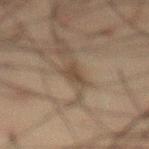{"biopsy_status": "not biopsied; imaged during a skin examination", "lesion_size": {"long_diameter_mm_approx": 3.0}, "patient": {"sex": "male", "age_approx": 60}, "image": {"source": "total-body photography crop", "field_of_view_mm": 15}, "automated_metrics": {"area_mm2_approx": 3.5, "eccentricity": 0.85, "shape_asymmetry": 0.45, "cielab_L": 34, "cielab_a": 11, "cielab_b": 21, "vs_skin_darker_L": 7.0, "vs_skin_contrast_norm": 7.0, "color_variation_0_10": 1.0, "peripheral_color_asymmetry": 0.5, "nevus_likeness_0_100": 15}, "site": "abdomen", "lighting": "cross-polarized"}